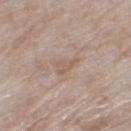Recorded during total-body skin imaging; not selected for excision or biopsy.